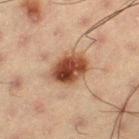Notes:
- follow-up · no biopsy performed (imaged during a skin exam)
- size · ≈4.5 mm
- image · total-body-photography crop, ~15 mm field of view
- site · the leg
- subject · male, aged around 55